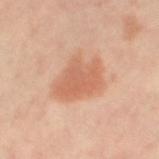Part of a total-body skin-imaging series; this lesion was reviewed on a skin check and was not flagged for biopsy.
A roughly 15 mm field-of-view crop from a total-body skin photograph.
A female patient, in their 50s.
The lesion is on the leg.